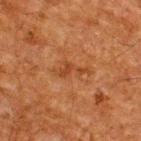Clinical impression:
The lesion was tiled from a total-body skin photograph and was not biopsied.
Image and clinical context:
Approximately 4 mm at its widest. Automated image analysis of the tile measured an eccentricity of roughly 0.9 and a symmetry-axis asymmetry near 0.6. It also reported an average lesion color of about L≈35 a*≈22 b*≈32 (CIELAB), roughly 6 lightness units darker than nearby skin, and a normalized border contrast of about 6. The analysis additionally found a nevus-likeness score of about 0/100 and a lesion-detection confidence of about 100/100. A 15 mm close-up extracted from a 3D total-body photography capture. Located on the back. A male subject, aged around 60. Imaged with cross-polarized lighting.